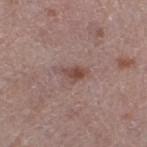<case>
<biopsy_status>not biopsied; imaged during a skin examination</biopsy_status>
<patient>
  <sex>female</sex>
  <age_approx>65</age_approx>
</patient>
<site>left thigh</site>
<image>
  <source>total-body photography crop</source>
  <field_of_view_mm>15</field_of_view_mm>
</image>
</case>